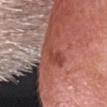follow-up: no biopsy performed (imaged during a skin exam)
anatomic site: the head or neck
imaging modality: ~15 mm tile from a whole-body skin photo
patient: male, aged 58–62